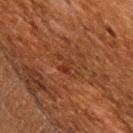Q: Was a biopsy performed?
A: no biopsy performed (imaged during a skin exam)
Q: Lesion size?
A: about 2.5 mm
Q: Who is the patient?
A: female, in their 50s
Q: Automated lesion metrics?
A: a footprint of about 4 mm², a shape eccentricity near 0.55, and two-axis asymmetry of about 0.35; border irregularity of about 4.5 on a 0–10 scale and peripheral color asymmetry of about 1; a nevus-likeness score of about 0/100 and lesion-presence confidence of about 95/100
Q: Illumination type?
A: cross-polarized
Q: What is the imaging modality?
A: 15 mm crop, total-body photography
Q: Lesion location?
A: the back